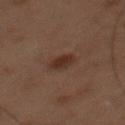Clinical impression: No biopsy was performed on this lesion — it was imaged during a full skin examination and was not determined to be concerning. Clinical summary: Approximately 3 mm at its widest. The subject is a male aged around 60. Cropped from a whole-body photographic skin survey; the tile spans about 15 mm. The lesion is on the mid back. Imaged with cross-polarized lighting.